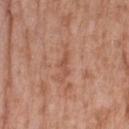Notes:
* follow-up · no biopsy performed (imaged during a skin exam)
* image source · ~15 mm tile from a whole-body skin photo
* automated metrics · a lesion area of about 3 mm² and an outline eccentricity of about 0.9 (0 = round, 1 = elongated); a color-variation rating of about 0/10 and radial color variation of about 0
* subject · female, about 75 years old
* anatomic site · the right forearm
* illumination · white-light